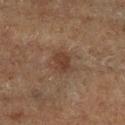workup=total-body-photography surveillance lesion; no biopsy | site=the leg | subject=male, in their mid-70s | image source=~15 mm crop, total-body skin-cancer survey.